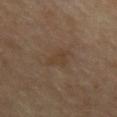Imaged with cross-polarized lighting. The lesion-visualizer software estimated an area of roughly 5 mm², an eccentricity of roughly 0.7, and a shape-asymmetry score of about 0.45 (0 = symmetric). The software also gave an automated nevus-likeness rating near 0 out of 100 and a detector confidence of about 100 out of 100 that the crop contains a lesion. The patient is a female about 50 years old. A close-up tile cropped from a whole-body skin photograph, about 15 mm across. From the front of the torso.